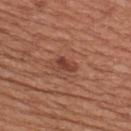Impression:
Imaged during a routine full-body skin examination; the lesion was not biopsied and no histopathology is available.
Background:
A male subject, in their mid- to late 60s. Imaged with white-light lighting. The lesion-visualizer software estimated a classifier nevus-likeness of about 65/100 and lesion-presence confidence of about 100/100. The lesion is located on the upper back. The lesion's longest dimension is about 2.5 mm. This image is a 15 mm lesion crop taken from a total-body photograph.